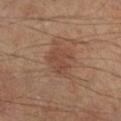Case summary:
– follow-up — catalogued during a skin exam; not biopsied
– automated metrics — an average lesion color of about L≈44 a*≈19 b*≈27 (CIELAB), about 7 CIELAB-L* units darker than the surrounding skin, and a lesion-to-skin contrast of about 5.5 (normalized; higher = more distinct); a border-irregularity index near 4.5/10, internal color variation of about 2.5 on a 0–10 scale, and radial color variation of about 1; an automated nevus-likeness rating near 40 out of 100 and a detector confidence of about 100 out of 100 that the crop contains a lesion
– lighting — cross-polarized illumination
– patient — male, aged 63 to 67
– body site — the right lower leg
– image — ~15 mm crop, total-body skin-cancer survey
– diameter — ~5.5 mm (longest diameter)